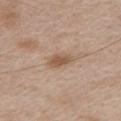{"biopsy_status": "not biopsied; imaged during a skin examination", "patient": {"sex": "male", "age_approx": 65}, "lesion_size": {"long_diameter_mm_approx": 3.0}, "lighting": "white-light", "image": {"source": "total-body photography crop", "field_of_view_mm": 15}, "site": "chest", "automated_metrics": {"area_mm2_approx": 4.5, "eccentricity": 0.8, "shape_asymmetry": 0.25, "cielab_L": 54, "cielab_a": 17, "cielab_b": 30, "vs_skin_darker_L": 10.0, "vs_skin_contrast_norm": 7.5, "border_irregularity_0_10": 2.5, "peripheral_color_asymmetry": 0.5, "nevus_likeness_0_100": 35, "lesion_detection_confidence_0_100": 100}}